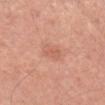workup — no biopsy performed (imaged during a skin exam)
automated metrics — a footprint of about 4.5 mm² and a shape eccentricity near 0.8; an average lesion color of about L≈61 a*≈26 b*≈31 (CIELAB) and roughly 7 lightness units darker than nearby skin
imaging modality — total-body-photography crop, ~15 mm field of view
location — the head or neck
patient — female, approximately 60 years of age
lighting — white-light
lesion size — ~3 mm (longest diameter)